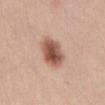  biopsy_status: not biopsied; imaged during a skin examination
  automated_metrics:
    cielab_L: 54
    cielab_a: 22
    cielab_b: 29
    vs_skin_darker_L: 16.0
    vs_skin_contrast_norm: 10.0
    lesion_detection_confidence_0_100: 100
  image:
    source: total-body photography crop
    field_of_view_mm: 15
  patient:
    sex: female
    age_approx: 35
  lesion_size:
    long_diameter_mm_approx: 4.0
  site: abdomen
  lighting: white-light